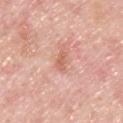Impression:
No biopsy was performed on this lesion — it was imaged during a full skin examination and was not determined to be concerning.
Context:
The lesion is on the upper back. Approximately 2.5 mm at its widest. The total-body-photography lesion software estimated a footprint of about 3 mm², an outline eccentricity of about 0.9 (0 = round, 1 = elongated), and a shape-asymmetry score of about 0.25 (0 = symmetric). The analysis additionally found an average lesion color of about L≈63 a*≈26 b*≈31 (CIELAB), about 9 CIELAB-L* units darker than the surrounding skin, and a normalized lesion–skin contrast near 6.5. The patient is a male in their mid- to late 40s. Cropped from a whole-body photographic skin survey; the tile spans about 15 mm.